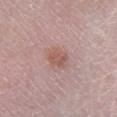The lesion was photographed on a routine skin check and not biopsied; there is no pathology result. Automated tile analysis of the lesion measured an area of roughly 5.5 mm² and an outline eccentricity of about 0.65 (0 = round, 1 = elongated). And it measured a mean CIELAB color near L≈56 a*≈21 b*≈24, roughly 8 lightness units darker than nearby skin, and a normalized border contrast of about 6.5. Cropped from a total-body skin-imaging series; the visible field is about 15 mm. Located on the right lower leg. A male subject aged around 50.